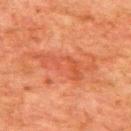biopsy status: total-body-photography surveillance lesion; no biopsy
location: the upper back
automated lesion analysis: a lesion area of about 14 mm², a shape eccentricity near 0.95, and a shape-asymmetry score of about 0.5 (0 = symmetric); a border-irregularity rating of about 7/10 and a within-lesion color-variation index near 3.5/10
patient: male, approximately 80 years of age
illumination: cross-polarized illumination
image source: ~15 mm crop, total-body skin-cancer survey